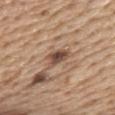biopsy status: imaged on a skin check; not biopsied | lesion size: ~3 mm (longest diameter) | illumination: white-light | acquisition: ~15 mm tile from a whole-body skin photo | location: the front of the torso | patient: male, aged approximately 70 | automated metrics: an average lesion color of about L≈49 a*≈18 b*≈28 (CIELAB) and a lesion-to-skin contrast of about 9 (normalized; higher = more distinct); border irregularity of about 3 on a 0–10 scale, internal color variation of about 4.5 on a 0–10 scale, and a peripheral color-asymmetry measure near 1; a lesion-detection confidence of about 100/100.